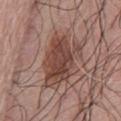Recorded during total-body skin imaging; not selected for excision or biopsy. An algorithmic analysis of the crop reported an average lesion color of about L≈44 a*≈20 b*≈24 (CIELAB) and roughly 11 lightness units darker than nearby skin. And it measured a border-irregularity rating of about 4.5/10, a within-lesion color-variation index near 5/10, and radial color variation of about 1.5. And it measured a classifier nevus-likeness of about 60/100. The lesion is located on the abdomen. The recorded lesion diameter is about 6.5 mm. A roughly 15 mm field-of-view crop from a total-body skin photograph. A female subject aged around 75.